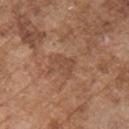The lesion was tiled from a total-body skin photograph and was not biopsied.
A male patient aged 73–77.
The lesion is on the front of the torso.
Measured at roughly 3 mm in maximum diameter.
The tile uses white-light illumination.
A 15 mm close-up extracted from a 3D total-body photography capture.
Automated image analysis of the tile measured an area of roughly 4.5 mm², an eccentricity of roughly 0.75, and a symmetry-axis asymmetry near 0.45. The analysis additionally found border irregularity of about 4.5 on a 0–10 scale, a color-variation rating of about 1/10, and a peripheral color-asymmetry measure near 0.5. The software also gave a classifier nevus-likeness of about 0/100 and a detector confidence of about 100 out of 100 that the crop contains a lesion.